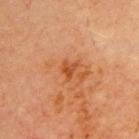The lesion was tiled from a total-body skin photograph and was not biopsied.
This is a cross-polarized tile.
A roughly 15 mm field-of-view crop from a total-body skin photograph.
On the front of the torso.
A male patient, in their 70s.